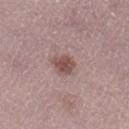The lesion was photographed on a routine skin check and not biopsied; there is no pathology result.
The lesion is on the right lower leg.
A female patient aged 48 to 52.
Measured at roughly 3 mm in maximum diameter.
A lesion tile, about 15 mm wide, cut from a 3D total-body photograph.
Automated image analysis of the tile measured an average lesion color of about L≈50 a*≈20 b*≈21 (CIELAB) and a lesion-to-skin contrast of about 8.5 (normalized; higher = more distinct). It also reported a nevus-likeness score of about 80/100.